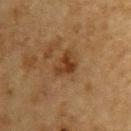Recorded during total-body skin imaging; not selected for excision or biopsy.
On the upper back.
A close-up tile cropped from a whole-body skin photograph, about 15 mm across.
A male subject in their mid-80s or older.